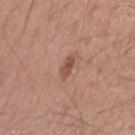<tbp_lesion>
  <biopsy_status>not biopsied; imaged during a skin examination</biopsy_status>
</tbp_lesion>